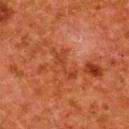Findings:
• notes — no biopsy performed (imaged during a skin exam)
• image source — ~15 mm crop, total-body skin-cancer survey
• image-analysis metrics — a symmetry-axis asymmetry near 0.5; a lesion color around L≈36 a*≈28 b*≈33 in CIELAB, roughly 6 lightness units darker than nearby skin, and a normalized border contrast of about 5.5; a detector confidence of about 95 out of 100 that the crop contains a lesion
• anatomic site — the upper back
• illumination — cross-polarized illumination
• subject — female, in their 50s